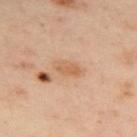Captured during whole-body skin photography for melanoma surveillance; the lesion was not biopsied. This is a cross-polarized tile. A female subject, aged 38 to 42. Located on the back. Approximately 3.5 mm at its widest. A region of skin cropped from a whole-body photographic capture, roughly 15 mm wide.